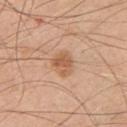Part of a total-body skin-imaging series; this lesion was reviewed on a skin check and was not flagged for biopsy. Captured under white-light illumination. Cropped from a total-body skin-imaging series; the visible field is about 15 mm. About 2.5 mm across. A male patient aged 48–52. The lesion is on the chest.